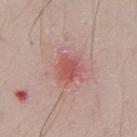Clinical impression: Imaged during a routine full-body skin examination; the lesion was not biopsied and no histopathology is available. Clinical summary: A male subject, about 50 years old. Imaged with white-light lighting. Approximately 3 mm at its widest. A 15 mm close-up tile from a total-body photography series done for melanoma screening. From the front of the torso.